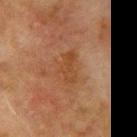Impression: Part of a total-body skin-imaging series; this lesion was reviewed on a skin check and was not flagged for biopsy. Background: The subject is a male aged 73–77. The lesion is on the arm. This image is a 15 mm lesion crop taken from a total-body photograph. Captured under cross-polarized illumination. The lesion-visualizer software estimated a border-irregularity index near 4/10, internal color variation of about 2.5 on a 0–10 scale, and radial color variation of about 1.